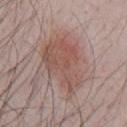Clinical impression:
The lesion was photographed on a routine skin check and not biopsied; there is no pathology result.
Image and clinical context:
The lesion is located on the chest. A male subject approximately 40 years of age. Measured at roughly 8 mm in maximum diameter. A 15 mm close-up tile from a total-body photography series done for melanoma screening.